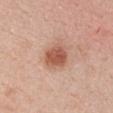Background:
A 15 mm crop from a total-body photograph taken for skin-cancer surveillance. A female subject, in their mid- to late 20s. Located on the chest.